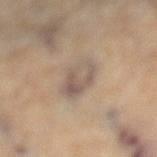Assessment: Recorded during total-body skin imaging; not selected for excision or biopsy. Image and clinical context: From the left lower leg. Approximately 4 mm at its widest. A female patient about 60 years old. Cropped from a total-body skin-imaging series; the visible field is about 15 mm. The tile uses cross-polarized illumination.